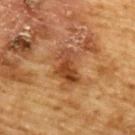follow-up: imaged on a skin check; not biopsied
body site: the upper back
TBP lesion metrics: an area of roughly 8 mm² and an outline eccentricity of about 0.8 (0 = round, 1 = elongated); border irregularity of about 4 on a 0–10 scale and radial color variation of about 2.5
image source: ~15 mm crop, total-body skin-cancer survey
patient: male, aged approximately 85 or older
size: ≈4 mm
lighting: cross-polarized